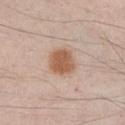Part of a total-body skin-imaging series; this lesion was reviewed on a skin check and was not flagged for biopsy.
Approximately 3 mm at its widest.
From the chest.
A male patient aged around 35.
Cropped from a total-body skin-imaging series; the visible field is about 15 mm.